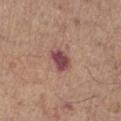Clinical impression: Imaged during a routine full-body skin examination; the lesion was not biopsied and no histopathology is available. Background: The lesion's longest dimension is about 3 mm. A 15 mm crop from a total-body photograph taken for skin-cancer surveillance. The lesion is located on the right forearm. Imaged with white-light lighting. The patient is a male aged around 65.